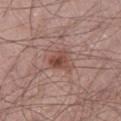Recorded during total-body skin imaging; not selected for excision or biopsy.
A lesion tile, about 15 mm wide, cut from a 3D total-body photograph.
Measured at roughly 3.5 mm in maximum diameter.
The tile uses white-light illumination.
A male subject roughly 65 years of age.
From the right thigh.